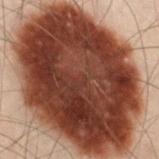Case summary:
– notes: no biopsy performed (imaged during a skin exam)
– imaging modality: ~15 mm crop, total-body skin-cancer survey
– tile lighting: cross-polarized
– patient: male, roughly 50 years of age
– site: the left leg
– image-analysis metrics: a footprint of about 120 mm², an eccentricity of roughly 0.7, and two-axis asymmetry of about 0.1; a lesion-to-skin contrast of about 19 (normalized; higher = more distinct); border irregularity of about 1.5 on a 0–10 scale and a within-lesion color-variation index near 6.5/10; a classifier nevus-likeness of about 75/100 and lesion-presence confidence of about 100/100
– lesion diameter: ≈15 mm